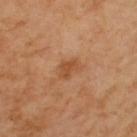Located on the left upper arm. This is a cross-polarized tile. A lesion tile, about 15 mm wide, cut from a 3D total-body photograph. An algorithmic analysis of the crop reported an area of roughly 3.5 mm², a shape eccentricity near 0.8, and a shape-asymmetry score of about 0.35 (0 = symmetric). And it measured a lesion color around L≈51 a*≈24 b*≈38 in CIELAB, about 8 CIELAB-L* units darker than the surrounding skin, and a lesion-to-skin contrast of about 6.5 (normalized; higher = more distinct). The software also gave a border-irregularity rating of about 3/10, internal color variation of about 1 on a 0–10 scale, and radial color variation of about 0.5. The patient is a female aged 53–57. The recorded lesion diameter is about 2.5 mm.